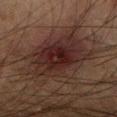<lesion>
  <biopsy_status>not biopsied; imaged during a skin examination</biopsy_status>
  <patient>
    <sex>male</sex>
    <age_approx>65</age_approx>
  </patient>
  <image>
    <source>total-body photography crop</source>
    <field_of_view_mm>15</field_of_view_mm>
  </image>
  <site>right forearm</site>
  <automated_metrics>
    <area_mm2_approx>40.0</area_mm2_approx>
    <eccentricity>0.55</eccentricity>
    <shape_asymmetry>0.25</shape_asymmetry>
    <cielab_L>21</cielab_L>
    <cielab_a>14</cielab_a>
    <cielab_b>17</cielab_b>
    <vs_skin_darker_L>7.0</vs_skin_darker_L>
    <vs_skin_contrast_norm>9.0</vs_skin_contrast_norm>
    <border_irregularity_0_10>4.0</border_irregularity_0_10>
    <color_variation_0_10>5.0</color_variation_0_10>
    <peripheral_color_asymmetry>1.5</peripheral_color_asymmetry>
  </automated_metrics>
  <lesion_size>
    <long_diameter_mm_approx>9.0</long_diameter_mm_approx>
  </lesion_size>
  <lighting>cross-polarized</lighting>
</lesion>